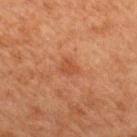Clinical impression: Part of a total-body skin-imaging series; this lesion was reviewed on a skin check and was not flagged for biopsy. Background: The total-body-photography lesion software estimated a shape eccentricity near 0.6 and a shape-asymmetry score of about 0.35 (0 = symmetric). Cropped from a total-body skin-imaging series; the visible field is about 15 mm. The lesion is located on the mid back. The recorded lesion diameter is about 2.5 mm. The patient is a female aged 38–42.